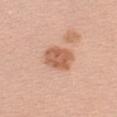| key | value |
|---|---|
| notes | total-body-photography surveillance lesion; no biopsy |